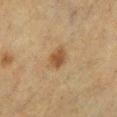Impression:
Captured during whole-body skin photography for melanoma surveillance; the lesion was not biopsied.
Background:
The recorded lesion diameter is about 2.5 mm. A female patient aged 53 to 57. Located on the left lower leg. The lesion-visualizer software estimated an area of roughly 5.5 mm² and two-axis asymmetry of about 0.15. It also reported border irregularity of about 1.5 on a 0–10 scale, internal color variation of about 3 on a 0–10 scale, and a peripheral color-asymmetry measure near 1. Imaged with cross-polarized lighting. This image is a 15 mm lesion crop taken from a total-body photograph.